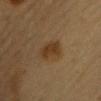Case summary:
- image · ~15 mm tile from a whole-body skin photo
- lesion size · ≈3 mm
- subject · female, aged around 30
- lighting · cross-polarized
- site · the left upper arm